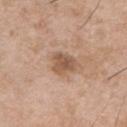  lesion_size:
    long_diameter_mm_approx: 3.5
  automated_metrics:
    cielab_L: 55
    cielab_a: 18
    cielab_b: 30
    vs_skin_darker_L: 11.0
    vs_skin_contrast_norm: 7.5
  lighting: white-light
  image:
    source: total-body photography crop
    field_of_view_mm: 15
  site: upper back
  patient:
    sex: male
    age_approx: 55